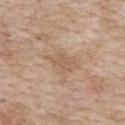Findings:
– follow-up: total-body-photography surveillance lesion; no biopsy
– lighting: white-light
– imaging modality: ~15 mm tile from a whole-body skin photo
– diameter: ≈4 mm
– site: the upper back
– subject: female, aged 73–77